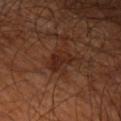Acquisition and patient details:
About 3 mm across. Located on the left upper arm. A male patient aged 43 to 47. This image is a 15 mm lesion crop taken from a total-body photograph. Imaged with cross-polarized lighting.